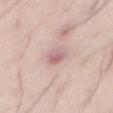workup: total-body-photography surveillance lesion; no biopsy | lesion size: ≈3 mm | patient: male, roughly 35 years of age | automated metrics: a lesion area of about 4.5 mm² and a shape eccentricity near 0.8; a normalized border contrast of about 7; a nevus-likeness score of about 0/100 and a lesion-detection confidence of about 95/100 | anatomic site: the abdomen | image: ~15 mm crop, total-body skin-cancer survey.